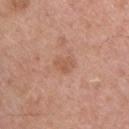Part of a total-body skin-imaging series; this lesion was reviewed on a skin check and was not flagged for biopsy. On the back. The subject is a male aged approximately 50. A lesion tile, about 15 mm wide, cut from a 3D total-body photograph.